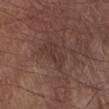  biopsy_status: not biopsied; imaged during a skin examination
  lighting: white-light
  automated_metrics:
    vs_skin_contrast_norm: 5.0
    border_irregularity_0_10: 4.5
    color_variation_0_10: 2.0
    nevus_likeness_0_100: 0
    lesion_detection_confidence_0_100: 90
  image:
    source: total-body photography crop
    field_of_view_mm: 15
  site: right lower leg
  patient:
    sex: male
    age_approx: 55